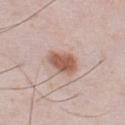The lesion was photographed on a routine skin check and not biopsied; there is no pathology result.
Approximately 4 mm at its widest.
A male patient, aged around 35.
A 15 mm close-up extracted from a 3D total-body photography capture.
The lesion is located on the front of the torso.
Captured under white-light illumination.